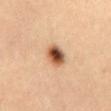Assessment:
Captured during whole-body skin photography for melanoma surveillance; the lesion was not biopsied.
Background:
A male patient aged approximately 20. A 15 mm crop from a total-body photograph taken for skin-cancer surveillance. The lesion is on the chest.